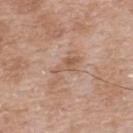{"biopsy_status": "not biopsied; imaged during a skin examination", "image": {"source": "total-body photography crop", "field_of_view_mm": 15}, "automated_metrics": {"cielab_L": 57, "cielab_a": 19, "cielab_b": 30, "vs_skin_darker_L": 7.0, "vs_skin_contrast_norm": 5.5, "nevus_likeness_0_100": 0, "lesion_detection_confidence_0_100": 100}, "lesion_size": {"long_diameter_mm_approx": 5.0}, "lighting": "white-light", "site": "back", "patient": {"sex": "male", "age_approx": 50}}